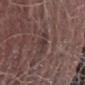Q: Was this lesion biopsied?
A: total-body-photography surveillance lesion; no biopsy
Q: Patient demographics?
A: male, aged approximately 40
Q: How large is the lesion?
A: ~2.5 mm (longest diameter)
Q: What lighting was used for the tile?
A: white-light
Q: What is the imaging modality?
A: ~15 mm tile from a whole-body skin photo
Q: Automated lesion metrics?
A: an area of roughly 4.5 mm² and a shape eccentricity near 0.4